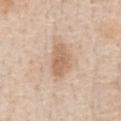follow-up: no biopsy performed (imaged during a skin exam)
imaging modality: total-body-photography crop, ~15 mm field of view
anatomic site: the chest
image-analysis metrics: an area of roughly 8.5 mm², an outline eccentricity of about 0.8 (0 = round, 1 = elongated), and a shape-asymmetry score of about 0.2 (0 = symmetric); a color-variation rating of about 2.5/10 and radial color variation of about 1; a nevus-likeness score of about 40/100 and a lesion-detection confidence of about 100/100
lesion diameter: about 4 mm
patient: male, aged 58 to 62
illumination: white-light illumination